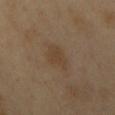This lesion was catalogued during total-body skin photography and was not selected for biopsy.
Approximately 4 mm at its widest.
Automated image analysis of the tile measured a footprint of about 6.5 mm², an outline eccentricity of about 0.8 (0 = round, 1 = elongated), and a shape-asymmetry score of about 0.35 (0 = symmetric). It also reported a detector confidence of about 100 out of 100 that the crop contains a lesion.
A region of skin cropped from a whole-body photographic capture, roughly 15 mm wide.
From the mid back.
Imaged with cross-polarized lighting.
The subject is a male aged 48 to 52.